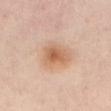Imaged during a routine full-body skin examination; the lesion was not biopsied and no histopathology is available. Captured under cross-polarized illumination. A female subject, aged approximately 50. A 15 mm crop from a total-body photograph taken for skin-cancer surveillance. The lesion is located on the abdomen. Automated tile analysis of the lesion measured a mean CIELAB color near L≈62 a*≈20 b*≈33, about 10 CIELAB-L* units darker than the surrounding skin, and a normalized border contrast of about 7.5. Measured at roughly 4 mm in maximum diameter.